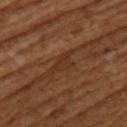notes: catalogued during a skin exam; not biopsied | diameter: about 2.5 mm | lighting: cross-polarized illumination | imaging modality: ~15 mm crop, total-body skin-cancer survey | location: the upper back | subject: female, roughly 55 years of age.